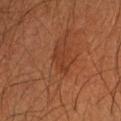follow-up = imaged on a skin check; not biopsied
diameter = ≈3 mm
patient = male, about 60 years old
lighting = cross-polarized illumination
imaging modality = total-body-photography crop, ~15 mm field of view
body site = the left forearm